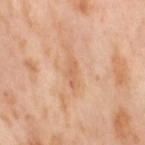Part of a total-body skin-imaging series; this lesion was reviewed on a skin check and was not flagged for biopsy. A female subject aged around 55. The lesion is on the right thigh. This image is a 15 mm lesion crop taken from a total-body photograph.